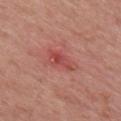Q: Was a biopsy performed?
A: imaged on a skin check; not biopsied
Q: How large is the lesion?
A: about 4 mm
Q: Where on the body is the lesion?
A: the chest
Q: How was this image acquired?
A: ~15 mm crop, total-body skin-cancer survey
Q: What are the patient's age and sex?
A: female, aged 63 to 67